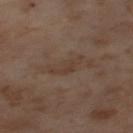Part of a total-body skin-imaging series; this lesion was reviewed on a skin check and was not flagged for biopsy. Imaged with cross-polarized lighting. Automated tile analysis of the lesion measured about 5 CIELAB-L* units darker than the surrounding skin and a normalized border contrast of about 5. It also reported border irregularity of about 6 on a 0–10 scale, internal color variation of about 1 on a 0–10 scale, and a peripheral color-asymmetry measure near 0.5. The analysis additionally found a nevus-likeness score of about 0/100. A 15 mm close-up extracted from a 3D total-body photography capture. The patient is a female in their mid-50s. On the left thigh.